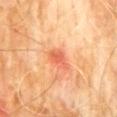| key | value |
|---|---|
| biopsy status | total-body-photography surveillance lesion; no biopsy |
| imaging modality | ~15 mm crop, total-body skin-cancer survey |
| tile lighting | cross-polarized illumination |
| patient | male, roughly 60 years of age |
| automated lesion analysis | internal color variation of about 2.5 on a 0–10 scale and peripheral color asymmetry of about 0.5 |
| location | the abdomen |
| diameter | ~3.5 mm (longest diameter) |